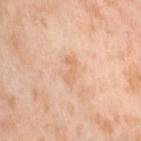The lesion was photographed on a routine skin check and not biopsied; there is no pathology result.
Approximately 3.5 mm at its widest.
On the right thigh.
A female patient, in their mid- to late 50s.
An algorithmic analysis of the crop reported an area of roughly 3.5 mm², an eccentricity of roughly 0.95, and two-axis asymmetry of about 0.5. It also reported a lesion color around L≈70 a*≈21 b*≈36 in CIELAB and roughly 7 lightness units darker than nearby skin. And it measured an automated nevus-likeness rating near 0 out of 100 and a lesion-detection confidence of about 100/100.
The tile uses cross-polarized illumination.
A 15 mm close-up extracted from a 3D total-body photography capture.